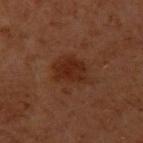  image:
    source: total-body photography crop
    field_of_view_mm: 15
  automated_metrics:
    eccentricity: 0.7
    shape_asymmetry: 0.3
    cielab_L: 25
    cielab_a: 21
    cielab_b: 26
    vs_skin_darker_L: 7.0
    vs_skin_contrast_norm: 7.5
  site: arm
  lesion_size:
    long_diameter_mm_approx: 4.5
  lighting: cross-polarized
  patient:
    sex: male
    age_approx: 60